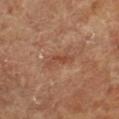follow-up: total-body-photography surveillance lesion; no biopsy | location: the chest | patient: female, aged approximately 80 | imaging modality: ~15 mm crop, total-body skin-cancer survey.